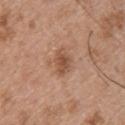Impression:
The lesion was photographed on a routine skin check and not biopsied; there is no pathology result.
Acquisition and patient details:
An algorithmic analysis of the crop reported a lesion area of about 5.5 mm², an outline eccentricity of about 0.75 (0 = round, 1 = elongated), and two-axis asymmetry of about 0.25. And it measured roughly 10 lightness units darker than nearby skin. The analysis additionally found an automated nevus-likeness rating near 20 out of 100. Measured at roughly 3.5 mm in maximum diameter. A 15 mm close-up extracted from a 3D total-body photography capture. A male patient aged approximately 50. On the upper back. This is a white-light tile.